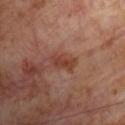– follow-up — catalogued during a skin exam; not biopsied
– anatomic site — the chest
– lighting — cross-polarized illumination
– TBP lesion metrics — a lesion area of about 4.5 mm², an eccentricity of roughly 0.85, and two-axis asymmetry of about 0.3; roughly 8 lightness units darker than nearby skin and a normalized border contrast of about 7; an automated nevus-likeness rating near 10 out of 100
– lesion size — about 3.5 mm
– patient — male, in their 70s
– acquisition — ~15 mm tile from a whole-body skin photo